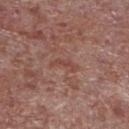lesion diameter — ~3.5 mm (longest diameter) | patient — male, aged around 55 | acquisition — ~15 mm crop, total-body skin-cancer survey | image-analysis metrics — an outline eccentricity of about 0.95 (0 = round, 1 = elongated) and a symmetry-axis asymmetry near 0.65; a mean CIELAB color near L≈45 a*≈23 b*≈25 and a normalized border contrast of about 5; a classifier nevus-likeness of about 0/100 and a detector confidence of about 80 out of 100 that the crop contains a lesion | location — the left lower leg.